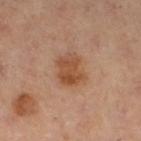Part of a total-body skin-imaging series; this lesion was reviewed on a skin check and was not flagged for biopsy.
On the right leg.
The recorded lesion diameter is about 3.5 mm.
The subject is a female aged approximately 60.
A roughly 15 mm field-of-view crop from a total-body skin photograph.
Captured under cross-polarized illumination.